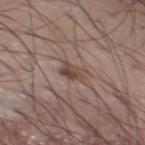The lesion was photographed on a routine skin check and not biopsied; there is no pathology result.
This image is a 15 mm lesion crop taken from a total-body photograph.
The recorded lesion diameter is about 3 mm.
Imaged with white-light lighting.
A male subject, aged 73 to 77.
The lesion is on the front of the torso.